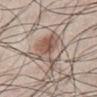Impression:
The lesion was tiled from a total-body skin photograph and was not biopsied.
Context:
A male subject in their mid- to late 40s. The lesion is on the chest. A roughly 15 mm field-of-view crop from a total-body skin photograph.